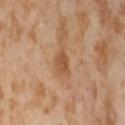– biopsy status · catalogued during a skin exam; not biopsied
– size · ~2.5 mm (longest diameter)
– subject · female, in their mid- to late 50s
– site · the right thigh
– acquisition · 15 mm crop, total-body photography
– lighting · cross-polarized